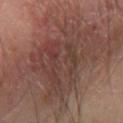Q: Was a biopsy performed?
A: no biopsy performed (imaged during a skin exam)
Q: What is the imaging modality?
A: ~15 mm tile from a whole-body skin photo
Q: How large is the lesion?
A: ~8.5 mm (longest diameter)
Q: Who is the patient?
A: male, roughly 70 years of age
Q: Automated lesion metrics?
A: a lesion area of about 38 mm² and an eccentricity of roughly 0.55; border irregularity of about 7.5 on a 0–10 scale, a color-variation rating of about 6.5/10, and radial color variation of about 2.5
Q: What lighting was used for the tile?
A: cross-polarized illumination
Q: What is the anatomic site?
A: the left forearm